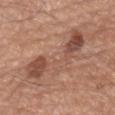Assessment:
Recorded during total-body skin imaging; not selected for excision or biopsy.
Image and clinical context:
The lesion is located on the left forearm. The subject is a male aged approximately 70. A close-up tile cropped from a whole-body skin photograph, about 15 mm across.